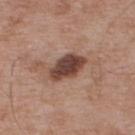biopsy status=catalogued during a skin exam; not biopsied | site=the upper back | acquisition=~15 mm crop, total-body skin-cancer survey | subject=male, aged around 55.